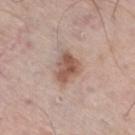<record>
<biopsy_status>not biopsied; imaged during a skin examination</biopsy_status>
<image>
  <source>total-body photography crop</source>
  <field_of_view_mm>15</field_of_view_mm>
</image>
<patient>
  <sex>male</sex>
  <age_approx>75</age_approx>
</patient>
<site>right thigh</site>
<lighting>white-light</lighting>
<lesion_size>
  <long_diameter_mm_approx>3.5</long_diameter_mm_approx>
</lesion_size>
</record>